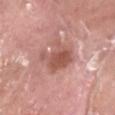Impression: The lesion was tiled from a total-body skin photograph and was not biopsied. Clinical summary: The lesion's longest dimension is about 5 mm. A male subject, aged 38 to 42. Cropped from a whole-body photographic skin survey; the tile spans about 15 mm. This is a white-light tile. The lesion is on the left lower leg.